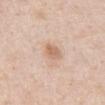biopsy_status: not biopsied; imaged during a skin examination
image:
  source: total-body photography crop
  field_of_view_mm: 15
lighting: white-light
lesion_size:
  long_diameter_mm_approx: 2.5
site: abdomen
patient:
  sex: male
  age_approx: 50
automated_metrics:
  area_mm2_approx: 4.5
  eccentricity: 0.6
  shape_asymmetry: 0.2
  cielab_L: 66
  cielab_a: 18
  cielab_b: 31
  vs_skin_contrast_norm: 6.5
  border_irregularity_0_10: 1.5
  color_variation_0_10: 2.5
  peripheral_color_asymmetry: 1.0